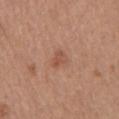illumination: white-light | imaging modality: 15 mm crop, total-body photography | body site: the chest | patient: male, approximately 65 years of age | image-analysis metrics: an area of roughly 3 mm², an outline eccentricity of about 0.8 (0 = round, 1 = elongated), and a shape-asymmetry score of about 0.4 (0 = symmetric); a lesion color around L≈52 a*≈23 b*≈30 in CIELAB, roughly 8 lightness units darker than nearby skin, and a lesion-to-skin contrast of about 5.5 (normalized; higher = more distinct); a border-irregularity index near 3.5/10, a within-lesion color-variation index near 1/10, and a peripheral color-asymmetry measure near 0.5 | size: ≈2.5 mm.